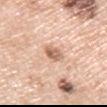Imaged during a routine full-body skin examination; the lesion was not biopsied and no histopathology is available. The lesion is on the arm. A roughly 15 mm field-of-view crop from a total-body skin photograph. A male patient aged 58–62.